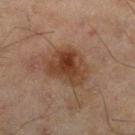biopsy status = no biopsy performed (imaged during a skin exam)
patient = male, aged around 45
location = the leg
diameter = ~5 mm (longest diameter)
acquisition = ~15 mm tile from a whole-body skin photo
TBP lesion metrics = a mean CIELAB color near L≈32 a*≈17 b*≈25, about 9 CIELAB-L* units darker than the surrounding skin, and a normalized border contrast of about 9; a border-irregularity index near 3/10, a within-lesion color-variation index near 5/10, and a peripheral color-asymmetry measure near 1.5; an automated nevus-likeness rating near 65 out of 100 and lesion-presence confidence of about 100/100
illumination = cross-polarized